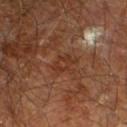| field | value |
|---|---|
| acquisition | total-body-photography crop, ~15 mm field of view |
| patient | male, roughly 60 years of age |
| illumination | cross-polarized illumination |
| size | ≈3 mm |
| automated lesion analysis | a lesion area of about 5 mm², an eccentricity of roughly 0.8, and a shape-asymmetry score of about 0.25 (0 = symmetric); a nevus-likeness score of about 0/100 |
| location | the right leg |